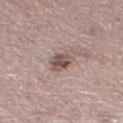Notes:
– notes: imaged on a skin check; not biopsied
– subject: female, aged around 50
– diameter: about 3 mm
– anatomic site: the right thigh
– illumination: white-light
– acquisition: total-body-photography crop, ~15 mm field of view
– image-analysis metrics: an average lesion color of about L≈51 a*≈17 b*≈21 (CIELAB), roughly 12 lightness units darker than nearby skin, and a normalized border contrast of about 8.5; border irregularity of about 2 on a 0–10 scale and a peripheral color-asymmetry measure near 1; a classifier nevus-likeness of about 40/100 and a lesion-detection confidence of about 100/100